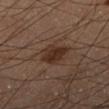{
  "biopsy_status": "not biopsied; imaged during a skin examination",
  "site": "right lower leg",
  "image": {
    "source": "total-body photography crop",
    "field_of_view_mm": 15
  },
  "patient": {
    "sex": "male",
    "age_approx": 60
  }
}